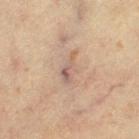Assessment: The lesion was photographed on a routine skin check and not biopsied; there is no pathology result. Clinical summary: A female subject, in their mid-60s. A 15 mm close-up extracted from a 3D total-body photography capture. The recorded lesion diameter is about 4 mm. Located on the left thigh. The total-body-photography lesion software estimated a symmetry-axis asymmetry near 0.4. The analysis additionally found a lesion color around L≈50 a*≈15 b*≈23 in CIELAB and about 7 CIELAB-L* units darker than the surrounding skin. And it measured border irregularity of about 4.5 on a 0–10 scale, internal color variation of about 4.5 on a 0–10 scale, and peripheral color asymmetry of about 1.5.